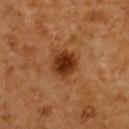This is a cross-polarized tile. A 15 mm crop from a total-body photograph taken for skin-cancer surveillance. A female subject about 55 years old. On the upper back. The lesion-visualizer software estimated an area of roughly 9.5 mm², a shape eccentricity near 0.35, and a shape-asymmetry score of about 0.2 (0 = symmetric). The analysis additionally found a mean CIELAB color near L≈36 a*≈25 b*≈36, a lesion–skin lightness drop of about 14, and a normalized border contrast of about 11. And it measured a border-irregularity rating of about 2/10, a color-variation rating of about 4.5/10, and radial color variation of about 1.5. And it measured a classifier nevus-likeness of about 100/100 and a detector confidence of about 100 out of 100 that the crop contains a lesion. Longest diameter approximately 3.5 mm.